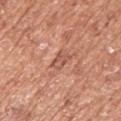This lesion was catalogued during total-body skin photography and was not selected for biopsy. A male subject roughly 75 years of age. About 3 mm across. A 15 mm crop from a total-body photograph taken for skin-cancer surveillance. The tile uses white-light illumination. Located on the left upper arm.